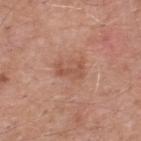{"biopsy_status": "not biopsied; imaged during a skin examination", "lighting": "white-light", "image": {"source": "total-body photography crop", "field_of_view_mm": 15}, "automated_metrics": {"area_mm2_approx": 4.0, "border_irregularity_0_10": 5.5, "color_variation_0_10": 0.0, "peripheral_color_asymmetry": 0.0}, "site": "upper back", "patient": {"sex": "male", "age_approx": 55}}